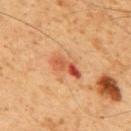The patient is a male approximately 60 years of age. On the upper back. A 15 mm close-up tile from a total-body photography series done for melanoma screening.